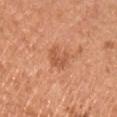The lesion was tiled from a total-body skin photograph and was not biopsied. A male subject, aged approximately 55. The lesion is on the chest. This image is a 15 mm lesion crop taken from a total-body photograph. An algorithmic analysis of the crop reported a footprint of about 5.5 mm², a shape eccentricity near 0.35, and a symmetry-axis asymmetry near 0.3. It also reported about 8 CIELAB-L* units darker than the surrounding skin and a lesion-to-skin contrast of about 5.5 (normalized; higher = more distinct). The software also gave a border-irregularity rating of about 4/10, internal color variation of about 2 on a 0–10 scale, and a peripheral color-asymmetry measure near 0.5. This is a white-light tile.